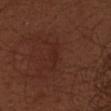From the upper back.
A close-up tile cropped from a whole-body skin photograph, about 15 mm across.
A female patient, about 30 years old.
The tile uses white-light illumination.